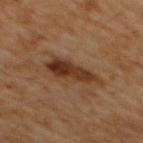Recorded during total-body skin imaging; not selected for excision or biopsy. This image is a 15 mm lesion crop taken from a total-body photograph. The lesion is located on the mid back. Imaged with cross-polarized lighting. An algorithmic analysis of the crop reported a footprint of about 11 mm², an outline eccentricity of about 0.9 (0 = round, 1 = elongated), and a shape-asymmetry score of about 0.3 (0 = symmetric). The software also gave an average lesion color of about L≈32 a*≈19 b*≈28 (CIELAB) and about 11 CIELAB-L* units darker than the surrounding skin. And it measured an automated nevus-likeness rating near 85 out of 100 and a detector confidence of about 100 out of 100 that the crop contains a lesion. A male subject, in their 60s.